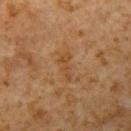The lesion was tiled from a total-body skin photograph and was not biopsied. The tile uses cross-polarized illumination. A roughly 15 mm field-of-view crop from a total-body skin photograph. About 3.5 mm across. A male patient aged 58–62. Automated image analysis of the tile measured an average lesion color of about L≈38 a*≈18 b*≈31 (CIELAB), about 5 CIELAB-L* units darker than the surrounding skin, and a lesion-to-skin contrast of about 5.5 (normalized; higher = more distinct). It also reported internal color variation of about 0 on a 0–10 scale and peripheral color asymmetry of about 0. The software also gave a lesion-detection confidence of about 100/100. From the left upper arm.